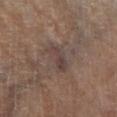Q: Is there a histopathology result?
A: no biopsy performed (imaged during a skin exam)
Q: Where on the body is the lesion?
A: the right lower leg
Q: What kind of image is this?
A: 15 mm crop, total-body photography
Q: What are the patient's age and sex?
A: female, aged 58 to 62